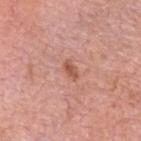Notes:
• automated lesion analysis — a lesion area of about 3 mm² and two-axis asymmetry of about 0.25; a mean CIELAB color near L≈55 a*≈26 b*≈30, a lesion–skin lightness drop of about 10, and a lesion-to-skin contrast of about 7 (normalized; higher = more distinct); a color-variation rating of about 2/10 and a peripheral color-asymmetry measure near 0.5
• lesion diameter — about 2.5 mm
• patient — male, roughly 80 years of age
• location — the head or neck
• illumination — white-light illumination
• image — ~15 mm tile from a whole-body skin photo